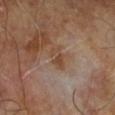workup: no biopsy performed (imaged during a skin exam) | patient: male, aged 63 to 67 | image: ~15 mm crop, total-body skin-cancer survey | diameter: ≈3 mm.